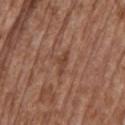The lesion was tiled from a total-body skin photograph and was not biopsied. A 15 mm crop from a total-body photograph taken for skin-cancer surveillance. Imaged with white-light lighting. The lesion is on the mid back. The recorded lesion diameter is about 3 mm. A male subject in their mid- to late 70s.